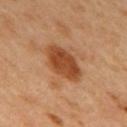notes: no biopsy performed (imaged during a skin exam) | lesion size: about 5 mm | illumination: cross-polarized | anatomic site: the mid back | subject: male, approximately 50 years of age | acquisition: total-body-photography crop, ~15 mm field of view.